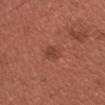The lesion was tiled from a total-body skin photograph and was not biopsied.
Captured under white-light illumination.
Located on the chest.
A male patient aged 33–37.
Longest diameter approximately 2.5 mm.
Cropped from a whole-body photographic skin survey; the tile spans about 15 mm.
An algorithmic analysis of the crop reported a footprint of about 3.5 mm² and an eccentricity of roughly 0.85. The software also gave a mean CIELAB color near L≈43 a*≈26 b*≈28, a lesion–skin lightness drop of about 7, and a normalized lesion–skin contrast near 6. It also reported a border-irregularity index near 2.5/10, a color-variation rating of about 2/10, and peripheral color asymmetry of about 1. The analysis additionally found an automated nevus-likeness rating near 10 out of 100.